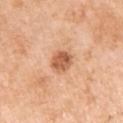Impression: No biopsy was performed on this lesion — it was imaged during a full skin examination and was not determined to be concerning. Acquisition and patient details: A female patient about 55 years old. Captured under white-light illumination. The lesion is located on the left upper arm. This image is a 15 mm lesion crop taken from a total-body photograph.